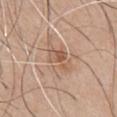Q: What are the patient's age and sex?
A: male, roughly 65 years of age
Q: How was this image acquired?
A: total-body-photography crop, ~15 mm field of view
Q: What is the anatomic site?
A: the chest